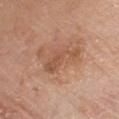Assessment:
No biopsy was performed on this lesion — it was imaged during a full skin examination and was not determined to be concerning.